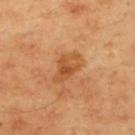<tbp_lesion>
<biopsy_status>not biopsied; imaged during a skin examination</biopsy_status>
<patient>
  <sex>male</sex>
  <age_approx>45</age_approx>
</patient>
<automated_metrics>
  <area_mm2_approx>7.0</area_mm2_approx>
  <eccentricity>0.8</eccentricity>
  <shape_asymmetry>0.3</shape_asymmetry>
  <nevus_likeness_0_100>5</nevus_likeness_0_100>
</automated_metrics>
<lesion_size>
  <long_diameter_mm_approx>4.0</long_diameter_mm_approx>
</lesion_size>
<image>
  <source>total-body photography crop</source>
  <field_of_view_mm>15</field_of_view_mm>
</image>
<site>upper back</site>
<lighting>cross-polarized</lighting>
</tbp_lesion>